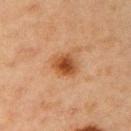Located on the right upper arm. The patient is a female approximately 45 years of age. A close-up tile cropped from a whole-body skin photograph, about 15 mm across. Imaged with cross-polarized lighting. Longest diameter approximately 3 mm.